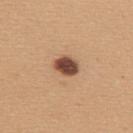  biopsy_status: not biopsied; imaged during a skin examination
  automated_metrics:
    vs_skin_contrast_norm: 13.5
    border_irregularity_0_10: 1.5
    color_variation_0_10: 4.0
    peripheral_color_asymmetry: 1.5
    nevus_likeness_0_100: 90
  site: upper back
  lesion_size:
    long_diameter_mm_approx: 3.0
  lighting: white-light
  patient:
    sex: female
    age_approx: 45
  image:
    source: total-body photography crop
    field_of_view_mm: 15